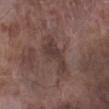This lesion was catalogued during total-body skin photography and was not selected for biopsy.
A close-up tile cropped from a whole-body skin photograph, about 15 mm across.
Automated image analysis of the tile measured an area of roughly 8.5 mm², a shape eccentricity near 0.9, and a shape-asymmetry score of about 0.5 (0 = symmetric). And it measured a mean CIELAB color near L≈37 a*≈16 b*≈19, roughly 8 lightness units darker than nearby skin, and a normalized lesion–skin contrast near 7. And it measured a border-irregularity index near 6/10, a within-lesion color-variation index near 1.5/10, and peripheral color asymmetry of about 0.5. The analysis additionally found a classifier nevus-likeness of about 0/100 and a lesion-detection confidence of about 95/100.
A male subject aged approximately 75.
From the left lower leg.
This is a white-light tile.
The lesion's longest dimension is about 5.5 mm.